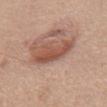{"biopsy_status": "not biopsied; imaged during a skin examination", "automated_metrics": {"border_irregularity_0_10": 4.5, "color_variation_0_10": 3.0, "peripheral_color_asymmetry": 1.0}, "lighting": "white-light", "patient": {"sex": "male", "age_approx": 70}, "lesion_size": {"long_diameter_mm_approx": 4.5}, "image": {"source": "total-body photography crop", "field_of_view_mm": 15}, "site": "chest"}